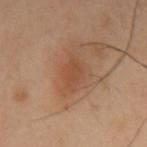Clinical impression: Captured during whole-body skin photography for melanoma surveillance; the lesion was not biopsied. Image and clinical context: The patient is a male aged 38–42. The recorded lesion diameter is about 3.5 mm. A 15 mm close-up tile from a total-body photography series done for melanoma screening. From the left upper arm.